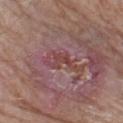– lighting — white-light illumination
– patient — male, aged 83 or over
– site — the left thigh
– acquisition — ~15 mm tile from a whole-body skin photo
– lesion size — about 5.5 mm
– TBP lesion metrics — a classifier nevus-likeness of about 0/100
– pathology — a nodular basal cell carcinoma